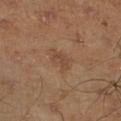Q: What is the anatomic site?
A: the right lower leg
Q: How large is the lesion?
A: ≈2.5 mm
Q: What are the patient's age and sex?
A: male, aged around 45
Q: Illumination type?
A: cross-polarized illumination
Q: What kind of image is this?
A: 15 mm crop, total-body photography
Q: Automated lesion metrics?
A: a within-lesion color-variation index near 2/10 and radial color variation of about 1